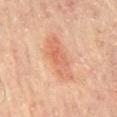Findings:
* notes: no biopsy performed (imaged during a skin exam)